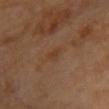Case summary:
– biopsy status: no biopsy performed (imaged during a skin exam)